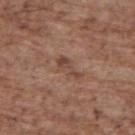Imaged during a routine full-body skin examination; the lesion was not biopsied and no histopathology is available. A close-up tile cropped from a whole-body skin photograph, about 15 mm across. The lesion is located on the upper back. A male patient, about 70 years old.